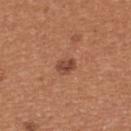Clinical impression:
The lesion was photographed on a routine skin check and not biopsied; there is no pathology result.
Context:
Approximately 3 mm at its widest. A female subject, aged 33–37. Cropped from a total-body skin-imaging series; the visible field is about 15 mm. Captured under white-light illumination. From the upper back.